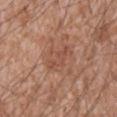notes: catalogued during a skin exam; not biopsied | acquisition: 15 mm crop, total-body photography | patient: male, aged approximately 60 | body site: the lower back.